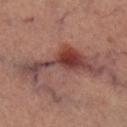Impression:
This lesion was catalogued during total-body skin photography and was not selected for biopsy.
Background:
Longest diameter approximately 10 mm. A female subject aged approximately 60. Located on the right lower leg. A region of skin cropped from a whole-body photographic capture, roughly 15 mm wide.